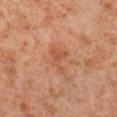workup: imaged on a skin check; not biopsied | size: ≈3.5 mm | illumination: cross-polarized | image source: ~15 mm tile from a whole-body skin photo | patient: male, about 55 years old | anatomic site: the leg.